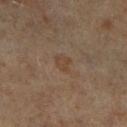Q: Was a biopsy performed?
A: no biopsy performed (imaged during a skin exam)
Q: Who is the patient?
A: female, roughly 65 years of age
Q: What is the imaging modality?
A: total-body-photography crop, ~15 mm field of view
Q: What is the lesion's diameter?
A: ~2.5 mm (longest diameter)
Q: Where on the body is the lesion?
A: the left leg